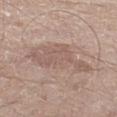Captured during whole-body skin photography for melanoma surveillance; the lesion was not biopsied.
A male subject, in their mid- to late 60s.
Imaged with white-light lighting.
From the left thigh.
The total-body-photography lesion software estimated an area of roughly 22 mm² and a shape eccentricity near 0.8. And it measured an automated nevus-likeness rating near 0 out of 100 and a lesion-detection confidence of about 95/100.
A lesion tile, about 15 mm wide, cut from a 3D total-body photograph.